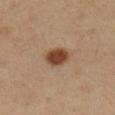workup = imaged on a skin check; not biopsied
anatomic site = the right lower leg
imaging modality = ~15 mm tile from a whole-body skin photo
subject = female, aged 33 to 37
size = about 3 mm